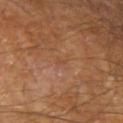follow-up: catalogued during a skin exam; not biopsied
TBP lesion metrics: an automated nevus-likeness rating near 0 out of 100 and a lesion-detection confidence of about 100/100
imaging modality: 15 mm crop, total-body photography
anatomic site: the left forearm
patient: male, about 70 years old